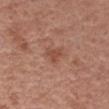The lesion is located on the right upper arm. A female patient, aged around 40. A region of skin cropped from a whole-body photographic capture, roughly 15 mm wide. An algorithmic analysis of the crop reported a mean CIELAB color near L≈49 a*≈24 b*≈29, roughly 8 lightness units darker than nearby skin, and a normalized lesion–skin contrast near 6. It also reported a color-variation rating of about 1.5/10 and radial color variation of about 0.5. Captured under white-light illumination. Longest diameter approximately 3 mm.